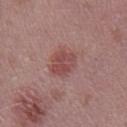Q: How was this image acquired?
A: total-body-photography crop, ~15 mm field of view
Q: Illumination type?
A: white-light illumination
Q: How large is the lesion?
A: ≈3 mm
Q: What is the anatomic site?
A: the right lower leg
Q: What are the patient's age and sex?
A: male, in their 40s